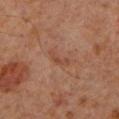image source = ~15 mm tile from a whole-body skin photo; subject = male, in their 60s; site = the left lower leg; illumination = cross-polarized illumination.